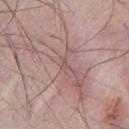Q: Is there a histopathology result?
A: no biopsy performed (imaged during a skin exam)
Q: What is the lesion's diameter?
A: ≈1.5 mm
Q: Automated lesion metrics?
A: an area of roughly 1 mm², a shape eccentricity near 0.85, and a shape-asymmetry score of about 0.6 (0 = symmetric); a mean CIELAB color near L≈54 a*≈19 b*≈20, a lesion–skin lightness drop of about 6, and a lesion-to-skin contrast of about 4 (normalized; higher = more distinct)
Q: What is the imaging modality?
A: total-body-photography crop, ~15 mm field of view
Q: Patient demographics?
A: male, about 40 years old
Q: Lesion location?
A: the chest
Q: How was the tile lit?
A: white-light illumination